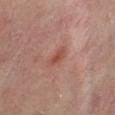workup: no biopsy performed (imaged during a skin exam)
body site: the abdomen
subject: female, aged around 65
imaging modality: ~15 mm tile from a whole-body skin photo
illumination: cross-polarized